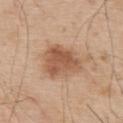Part of a total-body skin-imaging series; this lesion was reviewed on a skin check and was not flagged for biopsy.
Automated image analysis of the tile measured a lesion color around L≈55 a*≈21 b*≈33 in CIELAB and a normalized border contrast of about 8. The software also gave a border-irregularity rating of about 3/10 and radial color variation of about 1.5.
This image is a 15 mm lesion crop taken from a total-body photograph.
Longest diameter approximately 5 mm.
The lesion is on the upper back.
The subject is a male aged around 55.